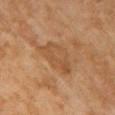{"biopsy_status": "not biopsied; imaged during a skin examination", "lighting": "cross-polarized", "image": {"source": "total-body photography crop", "field_of_view_mm": 15}, "patient": {"sex": "female", "age_approx": 60}, "lesion_size": {"long_diameter_mm_approx": 5.5}, "site": "chest"}